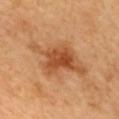notes — total-body-photography surveillance lesion; no biopsy
automated metrics — a mean CIELAB color near L≈54 a*≈26 b*≈41 and a lesion–skin lightness drop of about 12
subject — female, roughly 60 years of age
diameter — ≈8.5 mm
anatomic site — the upper back
tile lighting — cross-polarized illumination
imaging modality — ~15 mm tile from a whole-body skin photo